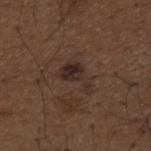Q: Was a biopsy performed?
A: imaged on a skin check; not biopsied
Q: Automated lesion metrics?
A: an area of roughly 6.5 mm², an eccentricity of roughly 0.8, and two-axis asymmetry of about 0.55; a nevus-likeness score of about 25/100
Q: How large is the lesion?
A: about 4 mm
Q: Lesion location?
A: the mid back
Q: What is the imaging modality?
A: total-body-photography crop, ~15 mm field of view
Q: Who is the patient?
A: male, aged 48–52